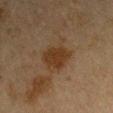Imaged during a routine full-body skin examination; the lesion was not biopsied and no histopathology is available.
The lesion is located on the right upper arm.
About 4 mm across.
A lesion tile, about 15 mm wide, cut from a 3D total-body photograph.
The tile uses cross-polarized illumination.
A male patient, aged approximately 65.
Automated tile analysis of the lesion measured a border-irregularity index near 3.5/10, internal color variation of about 3 on a 0–10 scale, and radial color variation of about 1. It also reported a detector confidence of about 100 out of 100 that the crop contains a lesion.